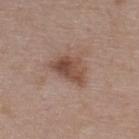follow-up=imaged on a skin check; not biopsied
lesion diameter=≈4.5 mm
patient=female, roughly 45 years of age
image-analysis metrics=an area of roughly 9 mm², an eccentricity of roughly 0.8, and two-axis asymmetry of about 0.35; border irregularity of about 3.5 on a 0–10 scale, internal color variation of about 6 on a 0–10 scale, and a peripheral color-asymmetry measure near 2.5; a lesion-detection confidence of about 100/100
illumination=white-light
anatomic site=the upper back
imaging modality=~15 mm crop, total-body skin-cancer survey